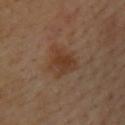follow-up=imaged on a skin check; not biopsied
TBP lesion metrics=an area of roughly 10 mm² and a shape eccentricity near 0.45; a lesion color around L≈37 a*≈18 b*≈29 in CIELAB, about 7 CIELAB-L* units darker than the surrounding skin, and a normalized border contrast of about 7; a classifier nevus-likeness of about 30/100 and a lesion-detection confidence of about 100/100
patient=male, aged around 40
location=the upper back
lesion diameter=~4 mm (longest diameter)
lighting=cross-polarized
image source=~15 mm tile from a whole-body skin photo